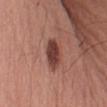Imaged during a routine full-body skin examination; the lesion was not biopsied and no histopathology is available. This is a white-light tile. The lesion is on the left forearm. A 15 mm crop from a total-body photograph taken for skin-cancer surveillance. A male patient aged around 60. The total-body-photography lesion software estimated a border-irregularity index near 2.5/10.